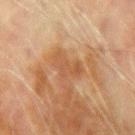Assessment: Captured during whole-body skin photography for melanoma surveillance; the lesion was not biopsied. Background: Automated image analysis of the tile measured an area of roughly 6.5 mm² and an eccentricity of roughly 0.85. The analysis additionally found a nevus-likeness score of about 0/100. A male subject about 70 years old. On the left upper arm. Measured at roughly 4 mm in maximum diameter. A 15 mm crop from a total-body photograph taken for skin-cancer surveillance.